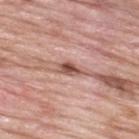| feature | finding |
|---|---|
| biopsy status | no biopsy performed (imaged during a skin exam) |
| location | the upper back |
| diameter | about 3 mm |
| subject | male, roughly 60 years of age |
| tile lighting | white-light |
| acquisition | 15 mm crop, total-body photography |